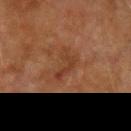Recorded during total-body skin imaging; not selected for excision or biopsy. The tile uses cross-polarized illumination. A region of skin cropped from a whole-body photographic capture, roughly 15 mm wide. The subject is a male in their mid-70s. Measured at roughly 4 mm in maximum diameter. The lesion is on the left upper arm. An algorithmic analysis of the crop reported a lesion area of about 5.5 mm², an eccentricity of roughly 0.85, and two-axis asymmetry of about 0.65. It also reported a lesion color around L≈31 a*≈20 b*≈27 in CIELAB, roughly 6 lightness units darker than nearby skin, and a lesion-to-skin contrast of about 5.5 (normalized; higher = more distinct). The software also gave a border-irregularity index near 8/10 and internal color variation of about 2 on a 0–10 scale. It also reported an automated nevus-likeness rating near 0 out of 100 and a detector confidence of about 100 out of 100 that the crop contains a lesion.